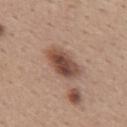The lesion was tiled from a total-body skin photograph and was not biopsied.
From the upper back.
A female patient roughly 40 years of age.
Cropped from a total-body skin-imaging series; the visible field is about 15 mm.